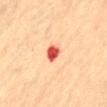The lesion was tiled from a total-body skin photograph and was not biopsied. The lesion-visualizer software estimated a normalized lesion–skin contrast near 12. The analysis additionally found border irregularity of about 2.5 on a 0–10 scale, internal color variation of about 3 on a 0–10 scale, and a peripheral color-asymmetry measure near 1. From the abdomen. About 2.5 mm across. A 15 mm crop from a total-body photograph taken for skin-cancer surveillance. A female subject in their 80s. This is a cross-polarized tile.